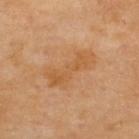Imaged during a routine full-body skin examination; the lesion was not biopsied and no histopathology is available.
Cropped from a whole-body photographic skin survey; the tile spans about 15 mm.
The lesion is on the upper back.
Imaged with cross-polarized lighting.
The subject is a male in their 70s.
The recorded lesion diameter is about 6 mm.